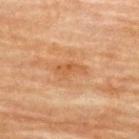biopsy_status: not biopsied; imaged during a skin examination
lesion_size:
  long_diameter_mm_approx: 3.5
site: back
image:
  source: total-body photography crop
  field_of_view_mm: 15
automated_metrics:
  border_irregularity_0_10: 4.0
  color_variation_0_10: 2.0
patient:
  sex: female
  age_approx: 80
lighting: cross-polarized